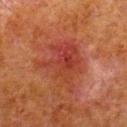workup: total-body-photography surveillance lesion; no biopsy | subject: male, aged around 80 | lesion size: ~6 mm (longest diameter) | illumination: cross-polarized | location: the right lower leg | image source: ~15 mm crop, total-body skin-cancer survey.